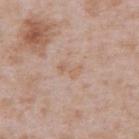Recorded during total-body skin imaging; not selected for excision or biopsy. The subject is a male in their mid-60s. Measured at roughly 3 mm in maximum diameter. The tile uses white-light illumination. The total-body-photography lesion software estimated a border-irregularity rating of about 4/10, a within-lesion color-variation index near 0.5/10, and a peripheral color-asymmetry measure near 0. The software also gave a classifier nevus-likeness of about 0/100. The lesion is located on the abdomen. Cropped from a whole-body photographic skin survey; the tile spans about 15 mm.